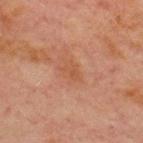This lesion was catalogued during total-body skin photography and was not selected for biopsy. The recorded lesion diameter is about 2.5 mm. The lesion is located on the chest. The patient is a male aged approximately 70. The lesion-visualizer software estimated a lesion area of about 3.5 mm² and two-axis asymmetry of about 0.3. The software also gave border irregularity of about 2.5 on a 0–10 scale, internal color variation of about 1.5 on a 0–10 scale, and radial color variation of about 0.5. It also reported a classifier nevus-likeness of about 0/100 and lesion-presence confidence of about 100/100. A region of skin cropped from a whole-body photographic capture, roughly 15 mm wide. Captured under cross-polarized illumination.